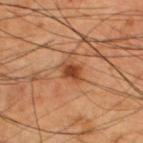The lesion was photographed on a routine skin check and not biopsied; there is no pathology result. The patient is a male approximately 60 years of age. A 15 mm close-up tile from a total-body photography series done for melanoma screening. The lesion is located on the upper back.